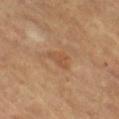biopsy status: imaged on a skin check; not biopsied
TBP lesion metrics: an area of roughly 4 mm², an outline eccentricity of about 0.75 (0 = round, 1 = elongated), and a shape-asymmetry score of about 0.5 (0 = symmetric); about 6 CIELAB-L* units darker than the surrounding skin; a border-irregularity index near 4.5/10, a color-variation rating of about 1/10, and peripheral color asymmetry of about 0.5
location: the right thigh
acquisition: ~15 mm crop, total-body skin-cancer survey
illumination: cross-polarized illumination
diameter: ~3 mm (longest diameter)
patient: female, aged approximately 70